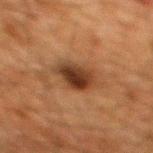tile lighting: cross-polarized
image source: ~15 mm tile from a whole-body skin photo
subject: male, about 60 years old
anatomic site: the back
size: ≈4 mm